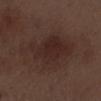Q: Was this lesion biopsied?
A: total-body-photography surveillance lesion; no biopsy
Q: Automated lesion metrics?
A: a shape eccentricity near 0.9 and a shape-asymmetry score of about 0.35 (0 = symmetric); a lesion color around L≈26 a*≈16 b*≈19 in CIELAB, a lesion–skin lightness drop of about 7, and a normalized border contrast of about 7.5; border irregularity of about 5 on a 0–10 scale, internal color variation of about 3 on a 0–10 scale, and a peripheral color-asymmetry measure near 1; a detector confidence of about 100 out of 100 that the crop contains a lesion
Q: What kind of image is this?
A: ~15 mm crop, total-body skin-cancer survey
Q: What are the patient's age and sex?
A: male, in their 70s
Q: Lesion location?
A: the right forearm
Q: What is the lesion's diameter?
A: ~10 mm (longest diameter)